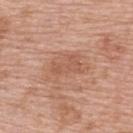Recorded during total-body skin imaging; not selected for excision or biopsy.
A 15 mm close-up tile from a total-body photography series done for melanoma screening.
Measured at roughly 5.5 mm in maximum diameter.
A female subject aged around 60.
The lesion is located on the upper back.